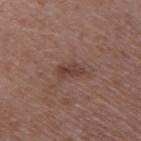workup — imaged on a skin check; not biopsied | acquisition — ~15 mm tile from a whole-body skin photo | size — ≈3 mm | tile lighting — white-light illumination | location — the right upper arm | patient — female, aged around 45.